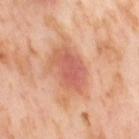follow-up = total-body-photography surveillance lesion; no biopsy | patient = female, roughly 55 years of age | anatomic site = the right thigh | imaging modality = ~15 mm crop, total-body skin-cancer survey.